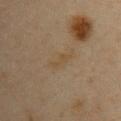Q: Was this lesion biopsied?
A: no biopsy performed (imaged during a skin exam)
Q: Patient demographics?
A: female, aged around 45
Q: Lesion location?
A: the right upper arm
Q: How was this image acquired?
A: total-body-photography crop, ~15 mm field of view
Q: How large is the lesion?
A: ≈2.5 mm